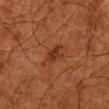The lesion was photographed on a routine skin check and not biopsied; there is no pathology result.